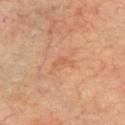Case summary:
- notes — catalogued during a skin exam; not biopsied
- acquisition — 15 mm crop, total-body photography
- subject — male, aged 68–72
- body site — the chest